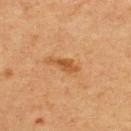Impression: Part of a total-body skin-imaging series; this lesion was reviewed on a skin check and was not flagged for biopsy. Image and clinical context: The patient is a male in their 60s. A 15 mm close-up tile from a total-body photography series done for melanoma screening. The tile uses cross-polarized illumination. An algorithmic analysis of the crop reported a lesion area of about 4 mm² and an eccentricity of roughly 0.9. It also reported a mean CIELAB color near L≈48 a*≈23 b*≈38, a lesion–skin lightness drop of about 9, and a normalized border contrast of about 7.5. The software also gave a classifier nevus-likeness of about 55/100 and a detector confidence of about 100 out of 100 that the crop contains a lesion. The recorded lesion diameter is about 3.5 mm. The lesion is located on the upper back.